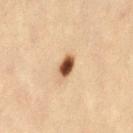Impression: Part of a total-body skin-imaging series; this lesion was reviewed on a skin check and was not flagged for biopsy. Clinical summary: A roughly 15 mm field-of-view crop from a total-body skin photograph. Imaged with cross-polarized lighting. The lesion is on the left thigh. The lesion's longest dimension is about 2.5 mm. A female patient aged approximately 45.